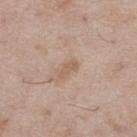The lesion was tiled from a total-body skin photograph and was not biopsied.
Imaged with white-light lighting.
A lesion tile, about 15 mm wide, cut from a 3D total-body photograph.
On the left thigh.
The lesion's longest dimension is about 2.5 mm.
A male patient, aged 48–52.